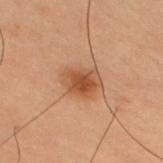Impression: No biopsy was performed on this lesion — it was imaged during a full skin examination and was not determined to be concerning. Background: A lesion tile, about 15 mm wide, cut from a 3D total-body photograph. This is a cross-polarized tile. A male patient, aged around 55. The lesion is located on the back. The total-body-photography lesion software estimated an average lesion color of about L≈42 a*≈20 b*≈30 (CIELAB) and about 9 CIELAB-L* units darker than the surrounding skin. The software also gave a border-irregularity rating of about 2/10, a within-lesion color-variation index near 4/10, and radial color variation of about 1. And it measured a classifier nevus-likeness of about 95/100 and lesion-presence confidence of about 100/100. About 4 mm across.